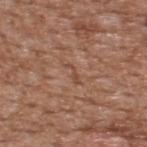Assessment: Imaged during a routine full-body skin examination; the lesion was not biopsied and no histopathology is available. Context: A 15 mm close-up extracted from a 3D total-body photography capture. Automated image analysis of the tile measured a lesion area of about 2 mm² and a shape-asymmetry score of about 0.45 (0 = symmetric). It also reported a mean CIELAB color near L≈48 a*≈21 b*≈30, a lesion–skin lightness drop of about 6, and a lesion-to-skin contrast of about 5 (normalized; higher = more distinct). And it measured a nevus-likeness score of about 0/100 and a lesion-detection confidence of about 65/100. A male patient, about 65 years old. Located on the upper back.